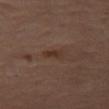The lesion was photographed on a routine skin check and not biopsied; there is no pathology result.
A close-up tile cropped from a whole-body skin photograph, about 15 mm across.
Located on the left thigh.
Approximately 3 mm at its widest.
A female subject roughly 65 years of age.